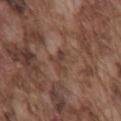Imaged during a routine full-body skin examination; the lesion was not biopsied and no histopathology is available.
Imaged with white-light lighting.
A male patient roughly 75 years of age.
On the front of the torso.
Longest diameter approximately 2.5 mm.
This image is a 15 mm lesion crop taken from a total-body photograph.
The total-body-photography lesion software estimated a lesion area of about 2.5 mm², a shape eccentricity near 0.9, and a symmetry-axis asymmetry near 0.6. The analysis additionally found internal color variation of about 0 on a 0–10 scale and radial color variation of about 0.